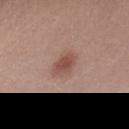biopsy status = imaged on a skin check; not biopsied
subject = male, roughly 30 years of age
imaging modality = total-body-photography crop, ~15 mm field of view
illumination = white-light